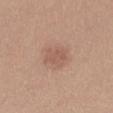Findings:
– biopsy status · total-body-photography surveillance lesion; no biopsy
– imaging modality · ~15 mm crop, total-body skin-cancer survey
– body site · the right thigh
– subject · female, approximately 30 years of age
– tile lighting · white-light illumination